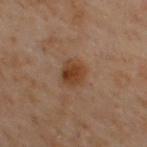  biopsy_status: not biopsied; imaged during a skin examination
  image:
    source: total-body photography crop
    field_of_view_mm: 15
  patient:
    sex: male
    age_approx: 50
  site: upper back